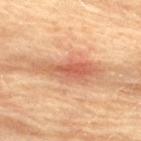workup: imaged on a skin check; not biopsied
site: the back
patient: female, about 80 years old
image: ~15 mm crop, total-body skin-cancer survey